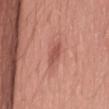Q: Was this lesion biopsied?
A: imaged on a skin check; not biopsied
Q: What lighting was used for the tile?
A: white-light
Q: What is the imaging modality?
A: total-body-photography crop, ~15 mm field of view
Q: Patient demographics?
A: female, aged around 40
Q: What is the anatomic site?
A: the lower back
Q: Lesion size?
A: about 3.5 mm